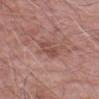Q: Was this lesion biopsied?
A: total-body-photography surveillance lesion; no biopsy
Q: What lighting was used for the tile?
A: white-light
Q: What did automated image analysis measure?
A: a lesion area of about 4.5 mm², an eccentricity of roughly 0.9, and a symmetry-axis asymmetry near 0.25
Q: How was this image acquired?
A: ~15 mm tile from a whole-body skin photo
Q: Where on the body is the lesion?
A: the right forearm
Q: What are the patient's age and sex?
A: male, about 75 years old
Q: Lesion size?
A: ~3.5 mm (longest diameter)